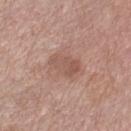The lesion was tiled from a total-body skin photograph and was not biopsied. A male patient, roughly 55 years of age. The lesion is on the chest. Cropped from a total-body skin-imaging series; the visible field is about 15 mm. Automated image analysis of the tile measured an average lesion color of about L≈54 a*≈20 b*≈26 (CIELAB), roughly 8 lightness units darker than nearby skin, and a normalized lesion–skin contrast near 6. And it measured a classifier nevus-likeness of about 5/100 and lesion-presence confidence of about 100/100.